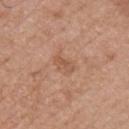<tbp_lesion>
<biopsy_status>not biopsied; imaged during a skin examination</biopsy_status>
<lesion_size>
  <long_diameter_mm_approx>2.5</long_diameter_mm_approx>
</lesion_size>
<site>chest</site>
<automated_metrics>
  <color_variation_0_10>2.0</color_variation_0_10>
  <peripheral_color_asymmetry>0.5</peripheral_color_asymmetry>
</automated_metrics>
<image>
  <source>total-body photography crop</source>
  <field_of_view_mm>15</field_of_view_mm>
</image>
<patient>
  <sex>male</sex>
  <age_approx>75</age_approx>
</patient>
<lighting>white-light</lighting>
</tbp_lesion>